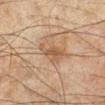Impression:
The lesion was tiled from a total-body skin photograph and was not biopsied.
Clinical summary:
A male patient, aged around 45. Longest diameter approximately 4 mm. The total-body-photography lesion software estimated an area of roughly 6.5 mm². And it measured about 8 CIELAB-L* units darker than the surrounding skin. The software also gave border irregularity of about 5 on a 0–10 scale, a within-lesion color-variation index near 2.5/10, and a peripheral color-asymmetry measure near 1. A region of skin cropped from a whole-body photographic capture, roughly 15 mm wide. Imaged with cross-polarized lighting. From the leg.